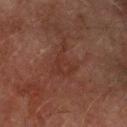{"biopsy_status": "not biopsied; imaged during a skin examination"}